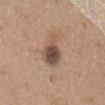A 15 mm close-up tile from a total-body photography series done for melanoma screening.
The subject is a male aged 63–67.
Measured at roughly 5 mm in maximum diameter.
This is a white-light tile.
On the mid back.